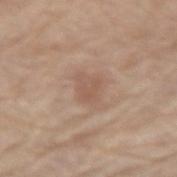biopsy_status: not biopsied; imaged during a skin examination
patient:
  sex: male
  age_approx: 70
image:
  source: total-body photography crop
  field_of_view_mm: 15
lighting: white-light
lesion_size:
  long_diameter_mm_approx: 3.0
site: right forearm